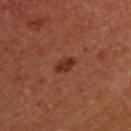Recorded during total-body skin imaging; not selected for excision or biopsy.
Automated image analysis of the tile measured a mean CIELAB color near L≈33 a*≈26 b*≈30, about 9 CIELAB-L* units darker than the surrounding skin, and a normalized lesion–skin contrast near 8. The software also gave a border-irregularity index near 1.5/10, a color-variation rating of about 2.5/10, and a peripheral color-asymmetry measure near 1.
The lesion is on the upper back.
A female subject aged approximately 40.
A roughly 15 mm field-of-view crop from a total-body skin photograph.
The lesion's longest dimension is about 2.5 mm.
Imaged with cross-polarized lighting.